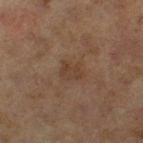Recorded during total-body skin imaging; not selected for excision or biopsy. A lesion tile, about 15 mm wide, cut from a 3D total-body photograph. A female subject about 60 years old. Located on the left thigh. Measured at roughly 2.5 mm in maximum diameter. Captured under cross-polarized illumination.